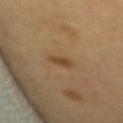Q: Is there a histopathology result?
A: no biopsy performed (imaged during a skin exam)
Q: Who is the patient?
A: female, aged 53 to 57
Q: What is the anatomic site?
A: the mid back
Q: What lighting was used for the tile?
A: cross-polarized illumination
Q: What is the lesion's diameter?
A: ~2 mm (longest diameter)
Q: What is the imaging modality?
A: total-body-photography crop, ~15 mm field of view